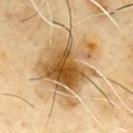biopsy status = catalogued during a skin exam; not biopsied | image source = 15 mm crop, total-body photography | site = the chest | size = about 8 mm | patient = male, about 65 years old | lighting = cross-polarized illumination.